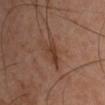workup: imaged on a skin check; not biopsied
diameter: ≈3 mm
tile lighting: cross-polarized illumination
subject: male, roughly 45 years of age
image-analysis metrics: a lesion area of about 5 mm² and an outline eccentricity of about 0.75 (0 = round, 1 = elongated); a lesion color around L≈35 a*≈19 b*≈26 in CIELAB, a lesion–skin lightness drop of about 7, and a lesion-to-skin contrast of about 6.5 (normalized; higher = more distinct)
image source: total-body-photography crop, ~15 mm field of view
anatomic site: the right upper arm